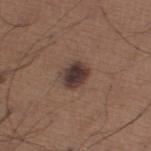The lesion was photographed on a routine skin check and not biopsied; there is no pathology result. The tile uses white-light illumination. A 15 mm close-up tile from a total-body photography series done for melanoma screening. A male subject, approximately 65 years of age. Longest diameter approximately 3 mm. From the right thigh.